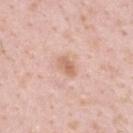Q: Was a biopsy performed?
A: no biopsy performed (imaged during a skin exam)
Q: How was the tile lit?
A: white-light
Q: What are the patient's age and sex?
A: male, aged approximately 35
Q: Lesion size?
A: about 2.5 mm
Q: How was this image acquired?
A: ~15 mm crop, total-body skin-cancer survey
Q: What is the anatomic site?
A: the upper back